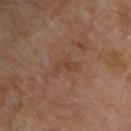follow-up: total-body-photography surveillance lesion; no biopsy | site: the chest | lighting: cross-polarized | subject: male, in their mid-50s | image: ~15 mm crop, total-body skin-cancer survey | lesion diameter: ≈3 mm.